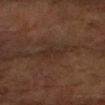{"biopsy_status": "not biopsied; imaged during a skin examination", "image": {"source": "total-body photography crop", "field_of_view_mm": 15}, "site": "right forearm", "patient": {"sex": "male", "age_approx": 75}}